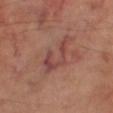No biopsy was performed on this lesion — it was imaged during a full skin examination and was not determined to be concerning.
Longest diameter approximately 6 mm.
The tile uses cross-polarized illumination.
A lesion tile, about 15 mm wide, cut from a 3D total-body photograph.
On the right thigh.
The subject is a male about 70 years old.